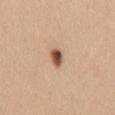Impression: No biopsy was performed on this lesion — it was imaged during a full skin examination and was not determined to be concerning. Background: The recorded lesion diameter is about 2.5 mm. This is a white-light tile. The subject is a female in their 30s. A roughly 15 mm field-of-view crop from a total-body skin photograph. From the back.